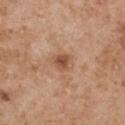<tbp_lesion>
<site>left upper arm</site>
<patient>
  <sex>male</sex>
  <age_approx>55</age_approx>
</patient>
<image>
  <source>total-body photography crop</source>
  <field_of_view_mm>15</field_of_view_mm>
</image>
</tbp_lesion>